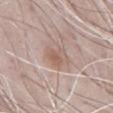The lesion was photographed on a routine skin check and not biopsied; there is no pathology result. The lesion's longest dimension is about 3.5 mm. A close-up tile cropped from a whole-body skin photograph, about 15 mm across. The tile uses white-light illumination. The total-body-photography lesion software estimated a border-irregularity rating of about 3/10 and radial color variation of about 1.5. The analysis additionally found a nevus-likeness score of about 5/100 and a detector confidence of about 100 out of 100 that the crop contains a lesion. Located on the abdomen. A male subject approximately 70 years of age.